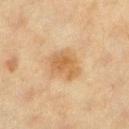biopsy_status: not biopsied; imaged during a skin examination
automated_metrics:
  area_mm2_approx: 9.5
  shape_asymmetry: 0.25
site: leg
lighting: cross-polarized
patient:
  sex: female
  age_approx: 40
image:
  source: total-body photography crop
  field_of_view_mm: 15
lesion_size:
  long_diameter_mm_approx: 4.5